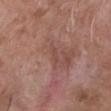Captured during whole-body skin photography for melanoma surveillance; the lesion was not biopsied.
Measured at roughly 2.5 mm in maximum diameter.
A close-up tile cropped from a whole-body skin photograph, about 15 mm across.
On the chest.
Captured under white-light illumination.
A male patient, aged around 50.